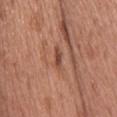{"image": {"source": "total-body photography crop", "field_of_view_mm": 15}, "lesion_size": {"long_diameter_mm_approx": 3.5}, "site": "upper back", "patient": {"sex": "male", "age_approx": 60}}